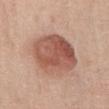Imaged during a routine full-body skin examination; the lesion was not biopsied and no histopathology is available. On the chest. A 15 mm close-up extracted from a 3D total-body photography capture. About 6.5 mm across. A male subject aged 68–72. Automated tile analysis of the lesion measured a lesion area of about 28 mm², an outline eccentricity of about 0.5 (0 = round, 1 = elongated), and a shape-asymmetry score of about 0.1 (0 = symmetric). It also reported a nevus-likeness score of about 75/100 and a detector confidence of about 100 out of 100 that the crop contains a lesion.